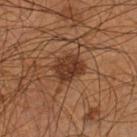Recorded during total-body skin imaging; not selected for excision or biopsy.
A 15 mm crop from a total-body photograph taken for skin-cancer surveillance.
A male patient, about 55 years old.
Located on the left lower leg.
An algorithmic analysis of the crop reported a footprint of about 9 mm², a shape eccentricity near 0.55, and a symmetry-axis asymmetry near 0.15. And it measured a mean CIELAB color near L≈32 a*≈19 b*≈28, about 10 CIELAB-L* units darker than the surrounding skin, and a normalized lesion–skin contrast near 9.5. And it measured border irregularity of about 1.5 on a 0–10 scale and a within-lesion color-variation index near 3/10. It also reported a detector confidence of about 100 out of 100 that the crop contains a lesion.
Longest diameter approximately 3.5 mm.